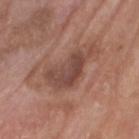The lesion was tiled from a total-body skin photograph and was not biopsied. A lesion tile, about 15 mm wide, cut from a 3D total-body photograph. The lesion is located on the head or neck. A male patient in their mid-70s. About 6 mm across.